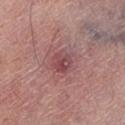Assessment:
Recorded during total-body skin imaging; not selected for excision or biopsy.
Clinical summary:
A lesion tile, about 15 mm wide, cut from a 3D total-body photograph. A male patient aged 53–57. Imaged with white-light lighting. The lesion is located on the left lower leg. Automated tile analysis of the lesion measured a footprint of about 5 mm² and a shape eccentricity near 0.4. The software also gave an average lesion color of about L≈46 a*≈26 b*≈19 (CIELAB), about 9 CIELAB-L* units darker than the surrounding skin, and a normalized border contrast of about 6.5. The recorded lesion diameter is about 2.5 mm.